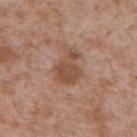notes: catalogued during a skin exam; not biopsied | automated lesion analysis: a border-irregularity index near 4/10, a color-variation rating of about 3/10, and radial color variation of about 1 | subject: male, in their mid- to late 40s | lesion diameter: ~4.5 mm (longest diameter) | imaging modality: ~15 mm tile from a whole-body skin photo | site: the upper back | tile lighting: white-light illumination.